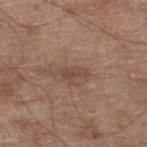<case>
<biopsy_status>not biopsied; imaged during a skin examination</biopsy_status>
<lesion_size>
  <long_diameter_mm_approx>3.0</long_diameter_mm_approx>
</lesion_size>
<site>left thigh</site>
<image>
  <source>total-body photography crop</source>
  <field_of_view_mm>15</field_of_view_mm>
</image>
<lighting>white-light</lighting>
<patient>
  <sex>male</sex>
  <age_approx>80</age_approx>
</patient>
</case>